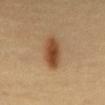Notes:
– illumination · cross-polarized illumination
– body site · the abdomen
– subject · female, aged around 45
– image source · ~15 mm tile from a whole-body skin photo